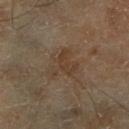Part of a total-body skin-imaging series; this lesion was reviewed on a skin check and was not flagged for biopsy.
A male subject about 70 years old.
A 15 mm crop from a total-body photograph taken for skin-cancer surveillance.
From the right lower leg.
Imaged with cross-polarized lighting.
The lesion-visualizer software estimated roughly 5 lightness units darker than nearby skin and a normalized border contrast of about 5.5. The software also gave border irregularity of about 7.5 on a 0–10 scale, a color-variation rating of about 2.5/10, and radial color variation of about 1. The software also gave an automated nevus-likeness rating near 0 out of 100 and a lesion-detection confidence of about 100/100.